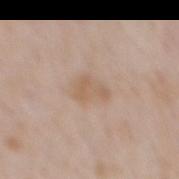Clinical impression: Recorded during total-body skin imaging; not selected for excision or biopsy. Image and clinical context: A male subject aged 63–67. A region of skin cropped from a whole-body photographic capture, roughly 15 mm wide. About 3 mm across. On the mid back. This is a white-light tile.